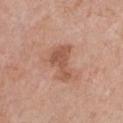The lesion's longest dimension is about 4.5 mm. The total-body-photography lesion software estimated a border-irregularity index near 6/10 and radial color variation of about 0.5. And it measured an automated nevus-likeness rating near 15 out of 100 and a lesion-detection confidence of about 100/100. The lesion is located on the chest. A female subject aged approximately 60. A 15 mm crop from a total-body photograph taken for skin-cancer surveillance. Captured under white-light illumination.